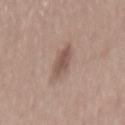- workup · total-body-photography surveillance lesion; no biopsy
- image-analysis metrics · a lesion color around L≈52 a*≈18 b*≈24 in CIELAB, about 11 CIELAB-L* units darker than the surrounding skin, and a normalized lesion–skin contrast near 7.5; a border-irregularity index near 4/10, a within-lesion color-variation index near 2.5/10, and a peripheral color-asymmetry measure near 1; a nevus-likeness score of about 70/100 and lesion-presence confidence of about 100/100
- illumination · white-light
- lesion diameter · ~4 mm (longest diameter)
- site · the mid back
- acquisition · ~15 mm crop, total-body skin-cancer survey
- patient · female, aged 53–57